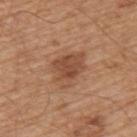<tbp_lesion>
  <biopsy_status>not biopsied; imaged during a skin examination</biopsy_status>
  <lesion_size>
    <long_diameter_mm_approx>4.5</long_diameter_mm_approx>
  </lesion_size>
  <image>
    <source>total-body photography crop</source>
    <field_of_view_mm>15</field_of_view_mm>
  </image>
  <patient>
    <sex>male</sex>
    <age_approx>65</age_approx>
  </patient>
  <automated_metrics>
    <area_mm2_approx>11.0</area_mm2_approx>
    <vs_skin_darker_L>9.0</vs_skin_darker_L>
    <vs_skin_contrast_norm>7.0</vs_skin_contrast_norm>
  </automated_metrics>
  <site>upper back</site>
  <lighting>white-light</lighting>
</tbp_lesion>